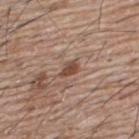notes: catalogued during a skin exam; not biopsied
patient: male, roughly 65 years of age
lighting: white-light illumination
lesion diameter: about 2.5 mm
acquisition: total-body-photography crop, ~15 mm field of view
location: the upper back